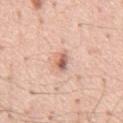Clinical impression:
The lesion was tiled from a total-body skin photograph and was not biopsied.
Background:
A male patient in their mid- to late 50s. From the chest. A 15 mm close-up extracted from a 3D total-body photography capture. Longest diameter approximately 2.5 mm. Automated tile analysis of the lesion measured an area of roughly 4 mm² and two-axis asymmetry of about 0.3. It also reported a border-irregularity index near 2.5/10 and peripheral color asymmetry of about 2.5. It also reported a nevus-likeness score of about 75/100 and a detector confidence of about 100 out of 100 that the crop contains a lesion. Imaged with white-light lighting.